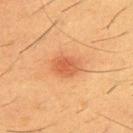Assessment:
This lesion was catalogued during total-body skin photography and was not selected for biopsy.
Acquisition and patient details:
The tile uses cross-polarized illumination. A male patient, aged around 55. The lesion is on the chest. About 3.5 mm across. A roughly 15 mm field-of-view crop from a total-body skin photograph.